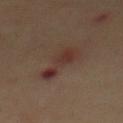biopsy status: total-body-photography surveillance lesion; no biopsy | subject: male, aged approximately 70 | TBP lesion metrics: a shape eccentricity near 0.9 and two-axis asymmetry of about 0.4; internal color variation of about 5.5 on a 0–10 scale and radial color variation of about 1.5; a classifier nevus-likeness of about 0/100 and a detector confidence of about 100 out of 100 that the crop contains a lesion | tile lighting: cross-polarized illumination | lesion size: ≈5.5 mm | acquisition: ~15 mm crop, total-body skin-cancer survey | location: the abdomen.